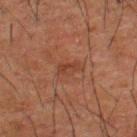| feature | finding |
|---|---|
| biopsy status | no biopsy performed (imaged during a skin exam) |
| imaging modality | ~15 mm tile from a whole-body skin photo |
| subject | male, about 50 years old |
| diameter | ≈3 mm |
| body site | the upper back |
| tile lighting | cross-polarized |